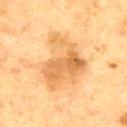Notes:
* biopsy status · catalogued during a skin exam; not biopsied
* lesion diameter · about 7 mm
* lighting · cross-polarized illumination
* acquisition · 15 mm crop, total-body photography
* subject · male, aged 63 to 67
* body site · the chest